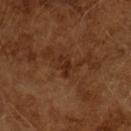Findings:
* biopsy status · imaged on a skin check; not biopsied
* tile lighting · cross-polarized
* TBP lesion metrics · an eccentricity of roughly 0.85 and a shape-asymmetry score of about 0.4 (0 = symmetric); a classifier nevus-likeness of about 0/100 and a detector confidence of about 100 out of 100 that the crop contains a lesion
* acquisition · total-body-photography crop, ~15 mm field of view
* patient · male, aged around 65